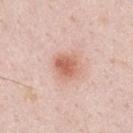Imaged during a routine full-body skin examination; the lesion was not biopsied and no histopathology is available. The recorded lesion diameter is about 2.5 mm. Captured under white-light illumination. On the left upper arm. Automated tile analysis of the lesion measured a footprint of about 6 mm², a shape eccentricity near 0.4, and two-axis asymmetry of about 0.2. The software also gave a border-irregularity rating of about 1.5/10, a color-variation rating of about 3/10, and radial color variation of about 1. A male patient approximately 35 years of age. A 15 mm close-up tile from a total-body photography series done for melanoma screening.